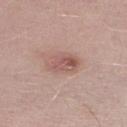Clinical impression:
Imaged during a routine full-body skin examination; the lesion was not biopsied and no histopathology is available.
Background:
Longest diameter approximately 4 mm. The lesion is located on the right lower leg. The total-body-photography lesion software estimated a lesion area of about 8.5 mm² and a shape eccentricity near 0.75. And it measured border irregularity of about 1.5 on a 0–10 scale, a color-variation rating of about 6/10, and radial color variation of about 2.5. And it measured a nevus-likeness score of about 65/100 and lesion-presence confidence of about 100/100. A male patient aged around 40. The tile uses white-light illumination. A close-up tile cropped from a whole-body skin photograph, about 15 mm across.